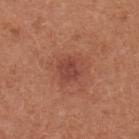Impression:
No biopsy was performed on this lesion — it was imaged during a full skin examination and was not determined to be concerning.
Background:
A roughly 15 mm field-of-view crop from a total-body skin photograph. The recorded lesion diameter is about 2.5 mm. The lesion-visualizer software estimated a border-irregularity index near 2/10 and internal color variation of about 2 on a 0–10 scale. The analysis additionally found a classifier nevus-likeness of about 5/100 and a lesion-detection confidence of about 100/100. Captured under white-light illumination. The lesion is located on the chest. A female patient, roughly 35 years of age.